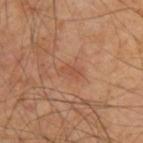The recorded lesion diameter is about 2.5 mm. This is a cross-polarized tile. A close-up tile cropped from a whole-body skin photograph, about 15 mm across. The patient is a male roughly 55 years of age. The lesion is on the upper back. Automated tile analysis of the lesion measured a lesion area of about 2.5 mm², a shape eccentricity near 0.85, and a symmetry-axis asymmetry near 0.3.